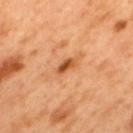Captured during whole-body skin photography for melanoma surveillance; the lesion was not biopsied. The lesion is located on the upper back. Cropped from a total-body skin-imaging series; the visible field is about 15 mm. This is a cross-polarized tile. Measured at roughly 3 mm in maximum diameter. A male subject aged around 50. Automated image analysis of the tile measured an automated nevus-likeness rating near 20 out of 100 and lesion-presence confidence of about 100/100.